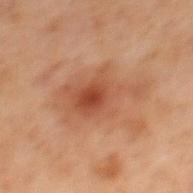Impression: Recorded during total-body skin imaging; not selected for excision or biopsy. Image and clinical context: The lesion's longest dimension is about 7.5 mm. From the mid back. This image is a 15 mm lesion crop taken from a total-body photograph. The tile uses cross-polarized illumination. A male patient, aged 68 to 72.